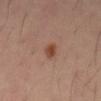The lesion was tiled from a total-body skin photograph and was not biopsied. This is a cross-polarized tile. The lesion is located on the front of the torso. Automated image analysis of the tile measured a within-lesion color-variation index near 2.5/10 and a peripheral color-asymmetry measure near 1. It also reported a nevus-likeness score of about 95/100. A 15 mm crop from a total-body photograph taken for skin-cancer surveillance. A male patient in their 40s.